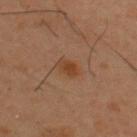Assessment:
The lesion was photographed on a routine skin check and not biopsied; there is no pathology result.
Image and clinical context:
Longest diameter approximately 3 mm. A male patient aged 38 to 42. On the upper back. Captured under cross-polarized illumination. A 15 mm close-up tile from a total-body photography series done for melanoma screening.